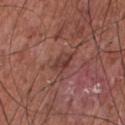Impression:
Imaged during a routine full-body skin examination; the lesion was not biopsied and no histopathology is available.
Image and clinical context:
A 15 mm close-up extracted from a 3D total-body photography capture. A male patient, aged 73–77. Located on the chest.